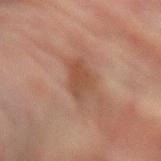Captured during whole-body skin photography for melanoma surveillance; the lesion was not biopsied. Longest diameter approximately 4 mm. The lesion is located on the left forearm. The tile uses cross-polarized illumination. The subject is a female roughly 80 years of age. This image is a 15 mm lesion crop taken from a total-body photograph.